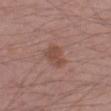{"biopsy_status": "not biopsied; imaged during a skin examination", "lighting": "white-light", "site": "leg", "patient": {"sex": "male", "age_approx": 55}, "automated_metrics": {"area_mm2_approx": 4.5, "eccentricity": 0.7, "cielab_L": 47, "cielab_a": 21, "cielab_b": 26, "vs_skin_darker_L": 8.0, "vs_skin_contrast_norm": 7.0}, "image": {"source": "total-body photography crop", "field_of_view_mm": 15}}